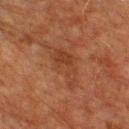Captured during whole-body skin photography for melanoma surveillance; the lesion was not biopsied.
A male subject aged around 80.
The lesion is on the chest.
A roughly 15 mm field-of-view crop from a total-body skin photograph.
Imaged with cross-polarized lighting.